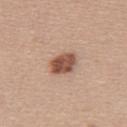| key | value |
|---|---|
| follow-up | total-body-photography surveillance lesion; no biopsy |
| body site | the upper back |
| image-analysis metrics | an outline eccentricity of about 0.7 (0 = round, 1 = elongated) and a symmetry-axis asymmetry near 0.15; a border-irregularity index near 1.5/10 and a color-variation rating of about 4/10 |
| subject | female, in their 40s |
| image | 15 mm crop, total-body photography |
| diameter | ~3.5 mm (longest diameter) |
| illumination | white-light |